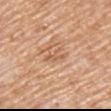Assessment:
The lesion was photographed on a routine skin check and not biopsied; there is no pathology result.
Clinical summary:
Approximately 3 mm at its widest. The lesion is located on the right upper arm. Imaged with white-light lighting. A male patient, aged 63–67. A region of skin cropped from a whole-body photographic capture, roughly 15 mm wide.